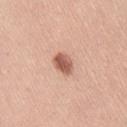Notes:
- workup · imaged on a skin check; not biopsied
- patient · female, in their 50s
- illumination · white-light
- site · the right thigh
- image-analysis metrics · a lesion area of about 4.5 mm², an outline eccentricity of about 0.75 (0 = round, 1 = elongated), and a symmetry-axis asymmetry near 0.15; radial color variation of about 1
- imaging modality · 15 mm crop, total-body photography
- lesion diameter · about 3 mm